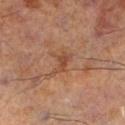{
  "biopsy_status": "not biopsied; imaged during a skin examination",
  "site": "left lower leg",
  "image": {
    "source": "total-body photography crop",
    "field_of_view_mm": 15
  },
  "lesion_size": {
    "long_diameter_mm_approx": 3.5
  },
  "patient": {
    "sex": "male",
    "age_approx": 70
  }
}